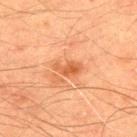The lesion was photographed on a routine skin check and not biopsied; there is no pathology result.
A male patient in their mid- to late 40s.
A close-up tile cropped from a whole-body skin photograph, about 15 mm across.
Imaged with cross-polarized lighting.
The lesion's longest dimension is about 3 mm.
The lesion is on the upper back.